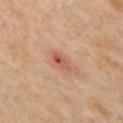No biopsy was performed on this lesion — it was imaged during a full skin examination and was not determined to be concerning.
The recorded lesion diameter is about 3 mm.
The lesion is located on the arm.
A 15 mm close-up extracted from a 3D total-body photography capture.
Automated tile analysis of the lesion measured a footprint of about 3.5 mm² and a symmetry-axis asymmetry near 0.2. It also reported peripheral color asymmetry of about 2. It also reported a classifier nevus-likeness of about 0/100 and a lesion-detection confidence of about 100/100.
The subject is a female aged approximately 50.
This is a cross-polarized tile.